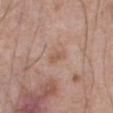Assessment:
Imaged during a routine full-body skin examination; the lesion was not biopsied and no histopathology is available.